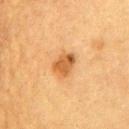A lesion tile, about 15 mm wide, cut from a 3D total-body photograph.
The lesion is located on the chest.
A female patient, aged 53–57.